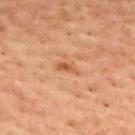Clinical impression: The lesion was photographed on a routine skin check and not biopsied; there is no pathology result. Clinical summary: The lesion is located on the back. Captured under cross-polarized illumination. A lesion tile, about 15 mm wide, cut from a 3D total-body photograph. Automated image analysis of the tile measured a nevus-likeness score of about 5/100 and a detector confidence of about 100 out of 100 that the crop contains a lesion. A male patient approximately 55 years of age. Longest diameter approximately 3 mm.